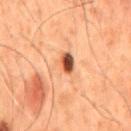Clinical impression: Imaged during a routine full-body skin examination; the lesion was not biopsied and no histopathology is available. Background: The tile uses cross-polarized illumination. Located on the mid back. The total-body-photography lesion software estimated a shape eccentricity near 0.6 and a symmetry-axis asymmetry near 0.45. The analysis additionally found border irregularity of about 5 on a 0–10 scale, a color-variation rating of about 10/10, and radial color variation of about 6.5. It also reported a nevus-likeness score of about 55/100. A 15 mm close-up extracted from a 3D total-body photography capture. The lesion's longest dimension is about 4 mm. A male patient, aged 48–52.